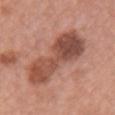| key | value |
|---|---|
| site | the chest |
| TBP lesion metrics | a lesion color around L≈50 a*≈24 b*≈28 in CIELAB, about 13 CIELAB-L* units darker than the surrounding skin, and a normalized lesion–skin contrast near 9; a border-irregularity index near 4/10, internal color variation of about 6.5 on a 0–10 scale, and a peripheral color-asymmetry measure near 2 |
| illumination | white-light |
| subject | female, aged 58–62 |
| acquisition | ~15 mm tile from a whole-body skin photo |